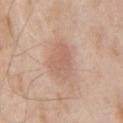Assessment: Captured during whole-body skin photography for melanoma surveillance; the lesion was not biopsied. Context: Automated tile analysis of the lesion measured roughly 8 lightness units darker than nearby skin and a lesion-to-skin contrast of about 5 (normalized; higher = more distinct). It also reported a border-irregularity rating of about 2/10 and radial color variation of about 1. The software also gave an automated nevus-likeness rating near 10 out of 100. A male patient in their 60s. About 4 mm across. A close-up tile cropped from a whole-body skin photograph, about 15 mm across. The lesion is located on the right upper arm. The tile uses white-light illumination.